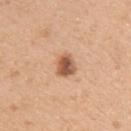* workup: catalogued during a skin exam; not biopsied